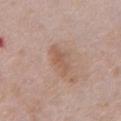Q: Was this lesion biopsied?
A: total-body-photography surveillance lesion; no biopsy
Q: What kind of image is this?
A: 15 mm crop, total-body photography
Q: What are the patient's age and sex?
A: female, in their mid-70s
Q: Lesion location?
A: the chest